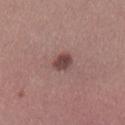biopsy_status: not biopsied; imaged during a skin examination
image:
  source: total-body photography crop
  field_of_view_mm: 15
lesion_size:
  long_diameter_mm_approx: 2.5
site: left lower leg
automated_metrics:
  area_mm2_approx: 4.0
  cielab_L: 43
  cielab_a: 20
  cielab_b: 19
  vs_skin_darker_L: 13.0
  border_irregularity_0_10: 1.5
  color_variation_0_10: 2.5
  peripheral_color_asymmetry: 1.0
lighting: white-light
patient:
  sex: male
  age_approx: 45